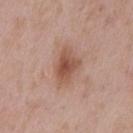Case summary:
* workup · catalogued during a skin exam; not biopsied
* acquisition · ~15 mm crop, total-body skin-cancer survey
* subject · male, in their mid-50s
* location · the mid back